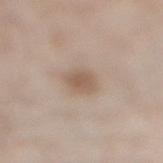workup: no biopsy performed (imaged during a skin exam)
image source: total-body-photography crop, ~15 mm field of view
diameter: ~3 mm (longest diameter)
site: the leg
lighting: white-light illumination
TBP lesion metrics: an outline eccentricity of about 0.5 (0 = round, 1 = elongated) and a shape-asymmetry score of about 0.2 (0 = symmetric); an average lesion color of about L≈57 a*≈15 b*≈27 (CIELAB), roughly 9 lightness units darker than nearby skin, and a lesion-to-skin contrast of about 6.5 (normalized; higher = more distinct); an automated nevus-likeness rating near 75 out of 100 and lesion-presence confidence of about 100/100
patient: male, roughly 60 years of age